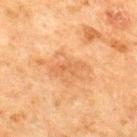follow-up: catalogued during a skin exam; not biopsied
patient: male, about 50 years old
anatomic site: the upper back
lesion size: ≈4 mm
lighting: cross-polarized illumination
automated lesion analysis: about 7 CIELAB-L* units darker than the surrounding skin and a normalized border contrast of about 5; a border-irregularity index near 5/10, a color-variation rating of about 0/10, and peripheral color asymmetry of about 0; an automated nevus-likeness rating near 0 out of 100 and lesion-presence confidence of about 100/100
acquisition: ~15 mm crop, total-body skin-cancer survey